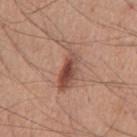Findings:
* illumination: white-light
* imaging modality: ~15 mm crop, total-body skin-cancer survey
* TBP lesion metrics: a lesion area of about 10 mm², an eccentricity of roughly 0.9, and a symmetry-axis asymmetry near 0.5; a lesion color around L≈50 a*≈22 b*≈27 in CIELAB, about 12 CIELAB-L* units darker than the surrounding skin, and a normalized lesion–skin contrast near 8.5; a border-irregularity rating of about 6/10, a within-lesion color-variation index near 7/10, and a peripheral color-asymmetry measure near 2.5; a nevus-likeness score of about 90/100 and lesion-presence confidence of about 100/100
* diameter: ~5.5 mm (longest diameter)
* body site: the abdomen
* patient: male, roughly 60 years of age